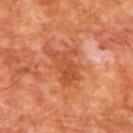No biopsy was performed on this lesion — it was imaged during a full skin examination and was not determined to be concerning.
Located on the upper back.
A roughly 15 mm field-of-view crop from a total-body skin photograph.
This is a cross-polarized tile.
A male subject roughly 65 years of age.
Measured at roughly 4.5 mm in maximum diameter.
Automated image analysis of the tile measured a footprint of about 8.5 mm², a shape eccentricity near 0.8, and a symmetry-axis asymmetry near 0.4. The software also gave a border-irregularity index near 6.5/10, internal color variation of about 2.5 on a 0–10 scale, and radial color variation of about 0.5.